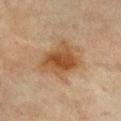Acquisition and patient details:
This is a cross-polarized tile. The lesion is located on the chest. A 15 mm close-up extracted from a 3D total-body photography capture. An algorithmic analysis of the crop reported a footprint of about 17 mm² and two-axis asymmetry of about 0.3. The software also gave an average lesion color of about L≈44 a*≈18 b*≈32 (CIELAB), about 10 CIELAB-L* units darker than the surrounding skin, and a normalized border contrast of about 9. And it measured a color-variation rating of about 5.5/10 and a peripheral color-asymmetry measure near 1.5. It also reported a classifier nevus-likeness of about 85/100 and a detector confidence of about 100 out of 100 that the crop contains a lesion. A female subject, in their mid- to late 60s. Measured at roughly 5.5 mm in maximum diameter.